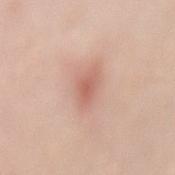Assessment:
Recorded during total-body skin imaging; not selected for excision or biopsy.
Background:
Automated image analysis of the tile measured an area of roughly 5 mm², an outline eccentricity of about 0.85 (0 = round, 1 = elongated), and a symmetry-axis asymmetry near 0.2. The software also gave an average lesion color of about L≈62 a*≈24 b*≈28 (CIELAB) and about 10 CIELAB-L* units darker than the surrounding skin. It also reported a border-irregularity rating of about 2.5/10 and a peripheral color-asymmetry measure near 0.5. Located on the back. A lesion tile, about 15 mm wide, cut from a 3D total-body photograph. A female subject aged approximately 50. The lesion's longest dimension is about 3.5 mm.